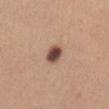Q: Was this lesion biopsied?
A: catalogued during a skin exam; not biopsied
Q: What lighting was used for the tile?
A: white-light
Q: What is the anatomic site?
A: the abdomen
Q: How was this image acquired?
A: ~15 mm tile from a whole-body skin photo
Q: What is the lesion's diameter?
A: ~3 mm (longest diameter)
Q: What are the patient's age and sex?
A: female, aged 43 to 47